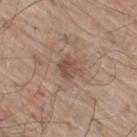Impression:
Imaged during a routine full-body skin examination; the lesion was not biopsied and no histopathology is available.
Clinical summary:
Located on the right thigh. A male patient, aged 68–72. A region of skin cropped from a whole-body photographic capture, roughly 15 mm wide. The total-body-photography lesion software estimated an area of roughly 5 mm² and an eccentricity of roughly 0.2. And it measured a lesion color around L≈49 a*≈18 b*≈27 in CIELAB. The analysis additionally found a border-irregularity index near 3/10, a color-variation rating of about 2/10, and a peripheral color-asymmetry measure near 0.5. The recorded lesion diameter is about 2.5 mm. The tile uses white-light illumination.